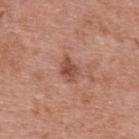workup — catalogued during a skin exam; not biopsied
acquisition — 15 mm crop, total-body photography
location — the back
illumination — white-light illumination
patient — female, roughly 70 years of age
lesion diameter — ≈2.5 mm